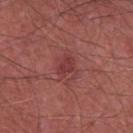Impression: Captured during whole-body skin photography for melanoma surveillance; the lesion was not biopsied. Context: A 15 mm close-up tile from a total-body photography series done for melanoma screening. Longest diameter approximately 2.5 mm. The tile uses white-light illumination. The lesion is located on the chest. A male subject roughly 65 years of age.